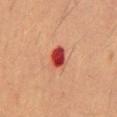Impression: The lesion was tiled from a total-body skin photograph and was not biopsied. Background: The subject is a male about 55 years old. The lesion is on the abdomen. The total-body-photography lesion software estimated a mean CIELAB color near L≈40 a*≈35 b*≈29, roughly 16 lightness units darker than nearby skin, and a lesion-to-skin contrast of about 12 (normalized; higher = more distinct). A 15 mm close-up extracted from a 3D total-body photography capture.